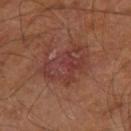biopsy status — total-body-photography surveillance lesion; no biopsy | anatomic site — the right leg | lighting — cross-polarized | patient — male, aged approximately 60 | acquisition — ~15 mm crop, total-body skin-cancer survey.